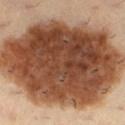Q: Was this lesion biopsied?
A: catalogued during a skin exam; not biopsied
Q: What are the patient's age and sex?
A: female, aged approximately 30
Q: What is the anatomic site?
A: the left lower leg
Q: How was the tile lit?
A: cross-polarized
Q: What did automated image analysis measure?
A: a footprint of about 130 mm² and an eccentricity of roughly 0.7; a lesion color around L≈44 a*≈20 b*≈30 in CIELAB, a lesion–skin lightness drop of about 21, and a normalized lesion–skin contrast near 14.5; a border-irregularity rating of about 2/10 and a color-variation rating of about 8/10
Q: How large is the lesion?
A: ~16 mm (longest diameter)
Q: How was this image acquired?
A: 15 mm crop, total-body photography